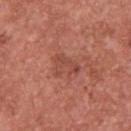{
  "biopsy_status": "not biopsied; imaged during a skin examination",
  "lesion_size": {
    "long_diameter_mm_approx": 3.5
  },
  "site": "chest",
  "image": {
    "source": "total-body photography crop",
    "field_of_view_mm": 15
  },
  "patient": {
    "sex": "male",
    "age_approx": 45
  },
  "lighting": "white-light"
}